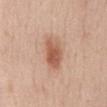| feature | finding |
|---|---|
| image source | 15 mm crop, total-body photography |
| anatomic site | the abdomen |
| subject | female, in their mid-40s |